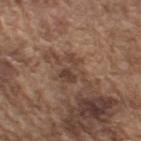The lesion was photographed on a routine skin check and not biopsied; there is no pathology result. The tile uses white-light illumination. An algorithmic analysis of the crop reported a footprint of about 5.5 mm², an outline eccentricity of about 0.75 (0 = round, 1 = elongated), and a symmetry-axis asymmetry near 0.5. The software also gave a lesion color around L≈41 a*≈17 b*≈25 in CIELAB. A male subject, in their mid- to late 70s. On the right upper arm. A lesion tile, about 15 mm wide, cut from a 3D total-body photograph.